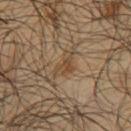The total-body-photography lesion software estimated a lesion color around L≈42 a*≈14 b*≈30 in CIELAB, roughly 6 lightness units darker than nearby skin, and a lesion-to-skin contrast of about 6 (normalized; higher = more distinct). And it measured a color-variation rating of about 3.5/10.
A male patient, aged approximately 65.
Approximately 3.5 mm at its widest.
Captured under cross-polarized illumination.
A lesion tile, about 15 mm wide, cut from a 3D total-body photograph.
On the upper back.